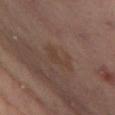Clinical impression:
Recorded during total-body skin imaging; not selected for excision or biopsy.
Acquisition and patient details:
The lesion is located on the left thigh. About 3 mm across. A female subject approximately 55 years of age. A region of skin cropped from a whole-body photographic capture, roughly 15 mm wide. Imaged with cross-polarized lighting.